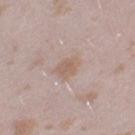Imaged during a routine full-body skin examination; the lesion was not biopsied and no histopathology is available.
Captured under white-light illumination.
Automated tile analysis of the lesion measured a mean CIELAB color near L≈60 a*≈15 b*≈25, roughly 7 lightness units darker than nearby skin, and a lesion-to-skin contrast of about 6 (normalized; higher = more distinct). It also reported border irregularity of about 2 on a 0–10 scale and a within-lesion color-variation index near 2/10. The analysis additionally found a classifier nevus-likeness of about 10/100.
Longest diameter approximately 3 mm.
A female patient, roughly 25 years of age.
The lesion is on the left thigh.
A roughly 15 mm field-of-view crop from a total-body skin photograph.